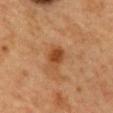biopsy status: catalogued during a skin exam; not biopsied | acquisition: ~15 mm tile from a whole-body skin photo | site: the mid back | illumination: cross-polarized | automated metrics: a footprint of about 5 mm², an outline eccentricity of about 0.6 (0 = round, 1 = elongated), and a symmetry-axis asymmetry near 0.2; a lesion color around L≈40 a*≈22 b*≈35 in CIELAB, about 10 CIELAB-L* units darker than the surrounding skin, and a normalized border contrast of about 8.5; border irregularity of about 2 on a 0–10 scale, a within-lesion color-variation index near 2.5/10, and radial color variation of about 0.5 | subject: female, aged 58 to 62.